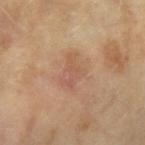| key | value |
|---|---|
| notes | no biopsy performed (imaged during a skin exam) |
| imaging modality | total-body-photography crop, ~15 mm field of view |
| subject | female, about 60 years old |
| anatomic site | the left forearm |